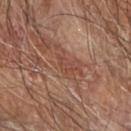Background: The tile uses cross-polarized illumination. Measured at roughly 3 mm in maximum diameter. Automated image analysis of the tile measured a footprint of about 3 mm² and two-axis asymmetry of about 0.3. The software also gave a mean CIELAB color near L≈44 a*≈22 b*≈28 and roughly 6 lightness units darker than nearby skin. The software also gave a border-irregularity index near 4/10 and radial color variation of about 0. A male patient aged 68–72. This image is a 15 mm lesion crop taken from a total-body photograph. From the arm.